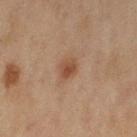Cropped from a whole-body photographic skin survey; the tile spans about 15 mm. About 2.5 mm across. The lesion is on the left upper arm. A female patient, aged 58 to 62. The total-body-photography lesion software estimated a lesion area of about 4 mm², an eccentricity of roughly 0.6, and a symmetry-axis asymmetry near 0.3. The tile uses cross-polarized illumination.